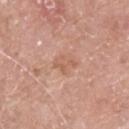Findings:
• notes · total-body-photography surveillance lesion; no biopsy
• patient · male, about 65 years old
• imaging modality · ~15 mm tile from a whole-body skin photo
• body site · the upper back